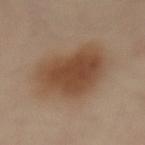Notes:
- biopsy status — imaged on a skin check; not biopsied
- image-analysis metrics — an eccentricity of roughly 0.5
- body site — the abdomen
- diameter — ≈7 mm
- imaging modality — ~15 mm crop, total-body skin-cancer survey
- tile lighting — cross-polarized illumination